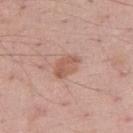Captured during whole-body skin photography for melanoma surveillance; the lesion was not biopsied. On the right upper arm. The lesion-visualizer software estimated a footprint of about 6.5 mm² and an outline eccentricity of about 0.7 (0 = round, 1 = elongated). A region of skin cropped from a whole-body photographic capture, roughly 15 mm wide. Longest diameter approximately 3.5 mm. A male patient, roughly 50 years of age. The tile uses white-light illumination.